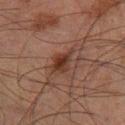The lesion was tiled from a total-body skin photograph and was not biopsied.
About 3.5 mm across.
A region of skin cropped from a whole-body photographic capture, roughly 15 mm wide.
A male patient about 70 years old.
The tile uses cross-polarized illumination.
An algorithmic analysis of the crop reported border irregularity of about 3 on a 0–10 scale and a color-variation rating of about 4/10.
The lesion is located on the right thigh.